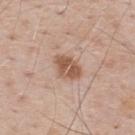follow-up: imaged on a skin check; not biopsied
body site: the back
patient: male, roughly 70 years of age
diameter: ≈3.5 mm
image: total-body-photography crop, ~15 mm field of view
illumination: white-light